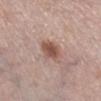{"patient": {"sex": "female", "age_approx": 65}, "image": {"source": "total-body photography crop", "field_of_view_mm": 15}, "site": "right lower leg"}